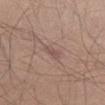Part of a total-body skin-imaging series; this lesion was reviewed on a skin check and was not flagged for biopsy. Located on the left thigh. The tile uses white-light illumination. Longest diameter approximately 2.5 mm. A male patient, aged around 45. A 15 mm close-up extracted from a 3D total-body photography capture.